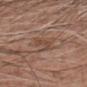notes: catalogued during a skin exam; not biopsied
subject: male, about 60 years old
lesion size: ~3.5 mm (longest diameter)
anatomic site: the left forearm
acquisition: total-body-photography crop, ~15 mm field of view